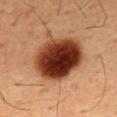notes: catalogued during a skin exam; not biopsied | subject: male, aged 53–57 | lesion diameter: about 7 mm | TBP lesion metrics: a footprint of about 28 mm², a shape eccentricity near 0.65, and a symmetry-axis asymmetry near 0.1; a border-irregularity rating of about 1/10, internal color variation of about 9.5 on a 0–10 scale, and peripheral color asymmetry of about 3 | location: the chest | imaging modality: total-body-photography crop, ~15 mm field of view.